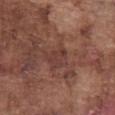follow-up = no biopsy performed (imaged during a skin exam); acquisition = ~15 mm crop, total-body skin-cancer survey; subject = male, approximately 75 years of age; diameter = about 2.5 mm; lighting = white-light illumination; location = the abdomen.